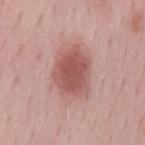Imaged during a routine full-body skin examination; the lesion was not biopsied and no histopathology is available. A male patient approximately 60 years of age. The tile uses white-light illumination. A close-up tile cropped from a whole-body skin photograph, about 15 mm across. The total-body-photography lesion software estimated an automated nevus-likeness rating near 95 out of 100 and a lesion-detection confidence of about 100/100. Longest diameter approximately 5.5 mm. On the mid back.